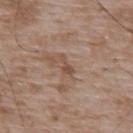Case summary:
- notes · imaged on a skin check; not biopsied
- patient · male, aged approximately 50
- automated metrics · a lesion area of about 2.5 mm², a shape eccentricity near 0.9, and a shape-asymmetry score of about 0.55 (0 = symmetric); a lesion color around L≈49 a*≈18 b*≈27 in CIELAB and a lesion–skin lightness drop of about 8; lesion-presence confidence of about 100/100
- acquisition · ~15 mm tile from a whole-body skin photo
- lighting · white-light illumination
- lesion size · ≈2.5 mm
- body site · the mid back